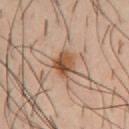Q: Was this lesion biopsied?
A: catalogued during a skin exam; not biopsied
Q: What lighting was used for the tile?
A: cross-polarized
Q: What is the imaging modality?
A: 15 mm crop, total-body photography
Q: What is the anatomic site?
A: the chest
Q: Automated lesion metrics?
A: a lesion area of about 7 mm², an eccentricity of roughly 0.6, and a symmetry-axis asymmetry near 0.2; a lesion color around L≈50 a*≈19 b*≈32 in CIELAB and a normalized lesion–skin contrast near 9.5
Q: Who is the patient?
A: male, approximately 40 years of age
Q: What is the lesion's diameter?
A: ≈3.5 mm